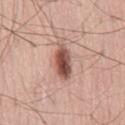Imaged during a routine full-body skin examination; the lesion was not biopsied and no histopathology is available. Approximately 4.5 mm at its widest. A 15 mm close-up extracted from a 3D total-body photography capture. The patient is a male aged 58 to 62. Automated tile analysis of the lesion measured an area of roughly 9 mm², a shape eccentricity near 0.85, and two-axis asymmetry of about 0.2. The analysis additionally found a nevus-likeness score of about 100/100 and a detector confidence of about 100 out of 100 that the crop contains a lesion. Located on the back. Imaged with white-light lighting.